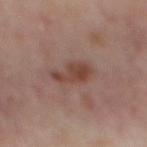Case summary:
– follow-up · imaged on a skin check; not biopsied
– location · the mid back
– acquisition · total-body-photography crop, ~15 mm field of view
– illumination · cross-polarized
– automated lesion analysis · a lesion color around L≈42 a*≈20 b*≈25 in CIELAB and a lesion-to-skin contrast of about 8 (normalized; higher = more distinct); a nevus-likeness score of about 50/100 and a lesion-detection confidence of about 100/100
– subject · aged 53 to 57
– lesion size · ~4 mm (longest diameter)